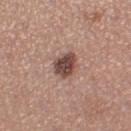The lesion was tiled from a total-body skin photograph and was not biopsied. A region of skin cropped from a whole-body photographic capture, roughly 15 mm wide. A female patient, about 30 years old. Approximately 3 mm at its widest. Automated image analysis of the tile measured an area of roughly 6.5 mm² and a shape-asymmetry score of about 0.25 (0 = symmetric). It also reported a lesion–skin lightness drop of about 16 and a normalized lesion–skin contrast near 11. It also reported a border-irregularity index near 2.5/10, a color-variation rating of about 4/10, and peripheral color asymmetry of about 1. The lesion is located on the leg. This is a white-light tile.